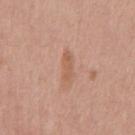Q: Is there a histopathology result?
A: total-body-photography surveillance lesion; no biopsy
Q: Lesion size?
A: ≈3.5 mm
Q: What lighting was used for the tile?
A: white-light
Q: What are the patient's age and sex?
A: male, aged 43–47
Q: What kind of image is this?
A: 15 mm crop, total-body photography
Q: Lesion location?
A: the mid back